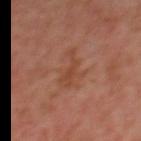Q: Is there a histopathology result?
A: imaged on a skin check; not biopsied
Q: Patient demographics?
A: male, aged 63 to 67
Q: What lighting was used for the tile?
A: cross-polarized
Q: Where on the body is the lesion?
A: the upper back
Q: What is the imaging modality?
A: 15 mm crop, total-body photography